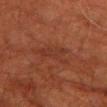Impression:
Imaged during a routine full-body skin examination; the lesion was not biopsied and no histopathology is available.
Background:
Imaged with cross-polarized lighting. From the left lower leg. Approximately 4.5 mm at its widest. Cropped from a total-body skin-imaging series; the visible field is about 15 mm. A male patient approximately 80 years of age.